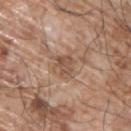{"biopsy_status": "not biopsied; imaged during a skin examination", "patient": {"sex": "male", "age_approx": 65}, "image": {"source": "total-body photography crop", "field_of_view_mm": 15}, "lighting": "white-light", "automated_metrics": {"color_variation_0_10": 2.5, "peripheral_color_asymmetry": 1.0, "lesion_detection_confidence_0_100": 95}, "lesion_size": {"long_diameter_mm_approx": 3.0}, "site": "upper back"}